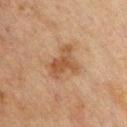{
  "biopsy_status": "not biopsied; imaged during a skin examination",
  "patient": {
    "sex": "male",
    "age_approx": 45
  },
  "site": "chest",
  "image": {
    "source": "total-body photography crop",
    "field_of_view_mm": 15
  },
  "lighting": "cross-polarized",
  "automated_metrics": {
    "eccentricity": 0.7,
    "shape_asymmetry": 0.5
  },
  "lesion_size": {
    "long_diameter_mm_approx": 4.5
  }
}